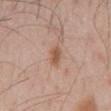{
  "biopsy_status": "not biopsied; imaged during a skin examination",
  "patient": {
    "sex": "male",
    "age_approx": 55
  },
  "lesion_size": {
    "long_diameter_mm_approx": 3.0
  },
  "automated_metrics": {
    "eccentricity": 0.85,
    "shape_asymmetry": 0.2,
    "cielab_L": 55,
    "cielab_a": 21,
    "cielab_b": 30,
    "border_irregularity_0_10": 2.5,
    "color_variation_0_10": 1.0,
    "peripheral_color_asymmetry": 0.5,
    "nevus_likeness_0_100": 65,
    "lesion_detection_confidence_0_100": 100
  },
  "site": "mid back",
  "image": {
    "source": "total-body photography crop",
    "field_of_view_mm": 15
  }
}